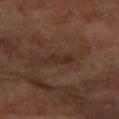Clinical impression:
Part of a total-body skin-imaging series; this lesion was reviewed on a skin check and was not flagged for biopsy.